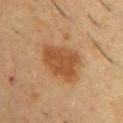This lesion was catalogued during total-body skin photography and was not selected for biopsy. The patient is a male approximately 55 years of age. Approximately 5 mm at its widest. The lesion is on the upper back. A 15 mm close-up tile from a total-body photography series done for melanoma screening.